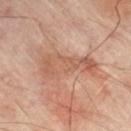follow-up — imaged on a skin check; not biopsied
location — the right thigh
patient — male, roughly 70 years of age
acquisition — total-body-photography crop, ~15 mm field of view